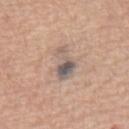• workup: total-body-photography surveillance lesion; no biopsy
• subject: female, in their 70s
• image source: total-body-photography crop, ~15 mm field of view
• illumination: white-light
• body site: the back
• automated lesion analysis: an average lesion color of about L≈56 a*≈12 b*≈20 (CIELAB), a lesion–skin lightness drop of about 11, and a normalized border contrast of about 9; a border-irregularity index near 5.5/10, a within-lesion color-variation index near 6/10, and peripheral color asymmetry of about 1.5
• diameter: about 4 mm